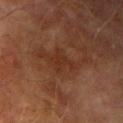notes: imaged on a skin check; not biopsied
tile lighting: cross-polarized
site: the right upper arm
subject: male, aged around 75
size: about 3.5 mm
image: total-body-photography crop, ~15 mm field of view